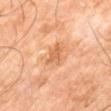Case summary:
- biopsy status — no biopsy performed (imaged during a skin exam)
- image — ~15 mm tile from a whole-body skin photo
- subject — male, roughly 60 years of age
- location — the right leg